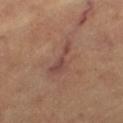| field | value |
|---|---|
| biopsy status | total-body-photography surveillance lesion; no biopsy |
| subject | aged approximately 60 |
| site | the left thigh |
| image | ~15 mm crop, total-body skin-cancer survey |
| size | about 4.5 mm |
| illumination | cross-polarized |